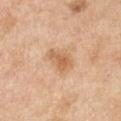workup = catalogued during a skin exam; not biopsied
imaging modality = ~15 mm crop, total-body skin-cancer survey
anatomic site = the left forearm
subject = female, in their 40s